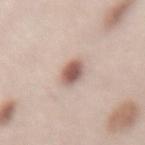  biopsy_status: not biopsied; imaged during a skin examination
  image:
    source: total-body photography crop
    field_of_view_mm: 15
  lighting: white-light
  site: lower back
  patient:
    sex: female
    age_approx: 65
  lesion_size:
    long_diameter_mm_approx: 3.0
  automated_metrics:
    vs_skin_darker_L: 16.0
    vs_skin_contrast_norm: 10.0
    nevus_likeness_0_100: 100